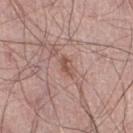location — the right lower leg; image source — 15 mm crop, total-body photography; patient — male, in their mid- to late 60s.